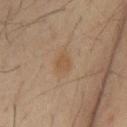<lesion>
<biopsy_status>not biopsied; imaged during a skin examination</biopsy_status>
<patient>
  <sex>male</sex>
  <age_approx>60</age_approx>
</patient>
<lighting>cross-polarized</lighting>
<image>
  <source>total-body photography crop</source>
  <field_of_view_mm>15</field_of_view_mm>
</image>
<site>mid back</site>
</lesion>